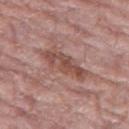{"biopsy_status": "not biopsied; imaged during a skin examination", "site": "leg", "lighting": "white-light", "image": {"source": "total-body photography crop", "field_of_view_mm": 15}, "lesion_size": {"long_diameter_mm_approx": 6.0}, "patient": {"sex": "female", "age_approx": 75}, "automated_metrics": {"shape_asymmetry": 0.25, "vs_skin_darker_L": 10.0, "vs_skin_contrast_norm": 7.5, "border_irregularity_0_10": 3.5, "color_variation_0_10": 4.0, "peripheral_color_asymmetry": 1.0, "nevus_likeness_0_100": 0, "lesion_detection_confidence_0_100": 75}}